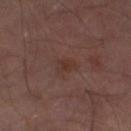biopsy_status: not biopsied; imaged during a skin examination
lighting: cross-polarized
patient:
  sex: male
  age_approx: 65
lesion_size:
  long_diameter_mm_approx: 2.5
automated_metrics:
  border_irregularity_0_10: 3.5
  color_variation_0_10: 1.5
  peripheral_color_asymmetry: 0.5
image:
  source: total-body photography crop
  field_of_view_mm: 15
site: leg